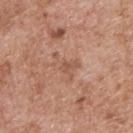Impression:
Captured during whole-body skin photography for melanoma surveillance; the lesion was not biopsied.
Acquisition and patient details:
On the upper back. The patient is a male approximately 60 years of age. Cropped from a whole-body photographic skin survey; the tile spans about 15 mm.